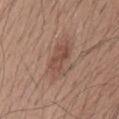follow-up — no biopsy performed (imaged during a skin exam)
location — the mid back
tile lighting — white-light
automated lesion analysis — a shape-asymmetry score of about 0.25 (0 = symmetric); a mean CIELAB color near L≈49 a*≈20 b*≈26 and a normalized border contrast of about 6; an automated nevus-likeness rating near 45 out of 100 and a detector confidence of about 100 out of 100 that the crop contains a lesion
image source — total-body-photography crop, ~15 mm field of view
subject — male, in their 40s